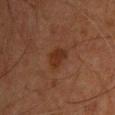biopsy status: imaged on a skin check; not biopsied | automated metrics: a mean CIELAB color near L≈25 a*≈19 b*≈25, a lesion–skin lightness drop of about 6, and a normalized lesion–skin contrast near 7.5; a border-irregularity index near 3/10; a nevus-likeness score of about 60/100 and a lesion-detection confidence of about 100/100 | lesion diameter: ≈2.5 mm | patient: male, about 45 years old | location: the chest | lighting: cross-polarized | acquisition: total-body-photography crop, ~15 mm field of view.